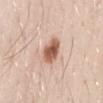<record>
  <biopsy_status>not biopsied; imaged during a skin examination</biopsy_status>
</record>